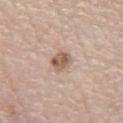– follow-up · no biopsy performed (imaged during a skin exam)
– diameter · ≈2.5 mm
– site · the front of the torso
– lighting · white-light
– patient · male, in their mid-70s
– TBP lesion metrics · a lesion area of about 5 mm², a shape eccentricity near 0.55, and a symmetry-axis asymmetry near 0.2; a nevus-likeness score of about 60/100
– image · 15 mm crop, total-body photography